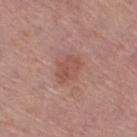Findings:
* biopsy status · no biopsy performed (imaged during a skin exam)
* illumination · white-light illumination
* site · the right thigh
* acquisition · ~15 mm crop, total-body skin-cancer survey
* automated metrics · a footprint of about 6.5 mm² and an eccentricity of roughly 0.75; an automated nevus-likeness rating near 15 out of 100 and a detector confidence of about 100 out of 100 that the crop contains a lesion
* patient · female, aged around 65
* diameter · ~3.5 mm (longest diameter)